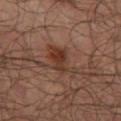image source = total-body-photography crop, ~15 mm field of view | anatomic site = the left thigh | lesion size = about 4.5 mm | subject = male, aged around 65 | tile lighting = cross-polarized.